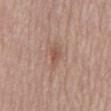workup = catalogued during a skin exam; not biopsied | acquisition = total-body-photography crop, ~15 mm field of view | subject = male, roughly 80 years of age | automated lesion analysis = two-axis asymmetry of about 0.35; border irregularity of about 3 on a 0–10 scale, internal color variation of about 1.5 on a 0–10 scale, and peripheral color asymmetry of about 0.5; an automated nevus-likeness rating near 0 out of 100 and a lesion-detection confidence of about 100/100 | anatomic site = the mid back.